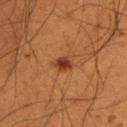biopsy_status: not biopsied; imaged during a skin examination
site: arm
patient:
  sex: male
  age_approx: 50
image:
  source: total-body photography crop
  field_of_view_mm: 15